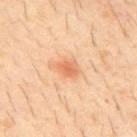workup: catalogued during a skin exam; not biopsied.